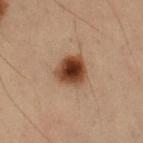Clinical impression: Recorded during total-body skin imaging; not selected for excision or biopsy. Acquisition and patient details: On the left forearm. The patient is a male approximately 55 years of age. A region of skin cropped from a whole-body photographic capture, roughly 15 mm wide. Automated image analysis of the tile measured a lesion area of about 9 mm², a shape eccentricity near 0.4, and two-axis asymmetry of about 0.25. And it measured internal color variation of about 5.5 on a 0–10 scale and peripheral color asymmetry of about 1. The analysis additionally found lesion-presence confidence of about 100/100. Imaged with cross-polarized lighting.